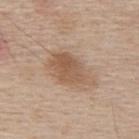The lesion was photographed on a routine skin check and not biopsied; there is no pathology result. Located on the upper back. A 15 mm crop from a total-body photograph taken for skin-cancer surveillance. A male patient, aged approximately 55.